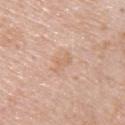| field | value |
|---|---|
| notes | catalogued during a skin exam; not biopsied |
| anatomic site | the upper back |
| patient | male, in their 50s |
| image source | total-body-photography crop, ~15 mm field of view |
| lesion diameter | ≈2.5 mm |
| tile lighting | white-light |